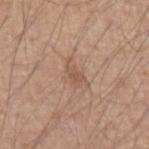biopsy status — catalogued during a skin exam; not biopsied | imaging modality — ~15 mm crop, total-body skin-cancer survey | patient — male, in their mid- to late 50s.